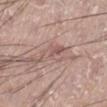Part of a total-body skin-imaging series; this lesion was reviewed on a skin check and was not flagged for biopsy. A male patient aged 48–52. A 15 mm close-up extracted from a 3D total-body photography capture. Located on the left lower leg. Imaged with white-light lighting.